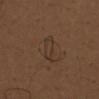Impression: Imaged during a routine full-body skin examination; the lesion was not biopsied and no histopathology is available. Background: About 4.5 mm across. A 15 mm close-up extracted from a 3D total-body photography capture. The lesion is on the front of the torso. Captured under white-light illumination. The total-body-photography lesion software estimated a lesion area of about 5.5 mm² and two-axis asymmetry of about 0.3. And it measured a mean CIELAB color near L≈34 a*≈14 b*≈24 and roughly 4 lightness units darker than nearby skin. The analysis additionally found lesion-presence confidence of about 95/100. The patient is a male about 70 years old.